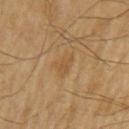Findings:
* workup — imaged on a skin check; not biopsied
* site — the left upper arm
* illumination — cross-polarized
* automated metrics — an area of roughly 3.5 mm² and a symmetry-axis asymmetry near 0.25; a lesion color around L≈48 a*≈16 b*≈36 in CIELAB; a border-irregularity index near 3/10, a within-lesion color-variation index near 1/10, and a peripheral color-asymmetry measure near 0.5; an automated nevus-likeness rating near 0 out of 100 and lesion-presence confidence of about 100/100
* imaging modality — ~15 mm crop, total-body skin-cancer survey
* subject — male, approximately 70 years of age
* lesion diameter — about 3 mm